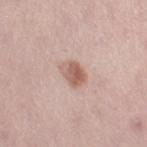{
  "site": "right thigh",
  "image": {
    "source": "total-body photography crop",
    "field_of_view_mm": 15
  },
  "patient": {
    "sex": "female",
    "age_approx": 30
  }
}